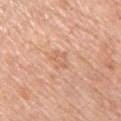  biopsy_status: not biopsied; imaged during a skin examination
  lighting: white-light
  image:
    source: total-body photography crop
    field_of_view_mm: 15
  patient:
    sex: male
    age_approx: 55
  site: upper back